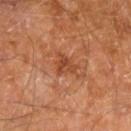Part of a total-body skin-imaging series; this lesion was reviewed on a skin check and was not flagged for biopsy. The lesion is on the right leg. Longest diameter approximately 2.5 mm. This image is a 15 mm lesion crop taken from a total-body photograph. A male subject aged 58 to 62. This is a cross-polarized tile.